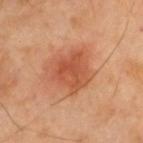<case>
  <biopsy_status>not biopsied; imaged during a skin examination</biopsy_status>
  <patient>
    <sex>male</sex>
    <age_approx>40</age_approx>
  </patient>
  <lighting>cross-polarized</lighting>
  <image>
    <source>total-body photography crop</source>
    <field_of_view_mm>15</field_of_view_mm>
  </image>
  <lesion_size>
    <long_diameter_mm_approx>4.5</long_diameter_mm_approx>
  </lesion_size>
  <automated_metrics>
    <area_mm2_approx>14.0</area_mm2_approx>
    <eccentricity>0.3</eccentricity>
    <color_variation_0_10>3.5</color_variation_0_10>
    <peripheral_color_asymmetry>1.0</peripheral_color_asymmetry>
    <lesion_detection_confidence_0_100>100</lesion_detection_confidence_0_100>
  </automated_metrics>
  <site>upper back</site>
</case>